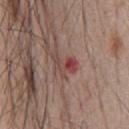Captured during whole-body skin photography for melanoma surveillance; the lesion was not biopsied. This is a white-light tile. The recorded lesion diameter is about 3.5 mm. A lesion tile, about 15 mm wide, cut from a 3D total-body photograph. A male subject, aged approximately 55.